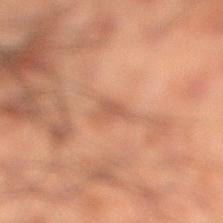| feature | finding |
|---|---|
| biopsy status | imaged on a skin check; not biopsied |
| location | the right lower leg |
| size | ~3 mm (longest diameter) |
| image source | 15 mm crop, total-body photography |
| illumination | cross-polarized illumination |
| patient | male, aged 48–52 |
| automated lesion analysis | a footprint of about 2.5 mm² and a shape eccentricity near 0.85; a lesion color around L≈43 a*≈19 b*≈26 in CIELAB, about 7 CIELAB-L* units darker than the surrounding skin, and a normalized border contrast of about 5.5; a border-irregularity rating of about 4.5/10 and a color-variation rating of about 0.5/10; an automated nevus-likeness rating near 0 out of 100 |